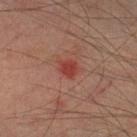Captured during whole-body skin photography for melanoma surveillance; the lesion was not biopsied. This is a cross-polarized tile. Approximately 2.5 mm at its widest. The subject is a male about 75 years old. Located on the left lower leg. A 15 mm close-up extracted from a 3D total-body photography capture.